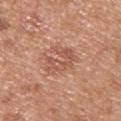Findings:
– lesion diameter — about 3.5 mm
– illumination — white-light
– site — the upper back
– imaging modality — ~15 mm crop, total-body skin-cancer survey
– subject — male, aged 28–32
– image-analysis metrics — a footprint of about 8.5 mm²; a within-lesion color-variation index near 3/10 and radial color variation of about 1; a nevus-likeness score of about 0/100 and lesion-presence confidence of about 100/100